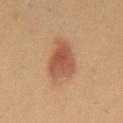| key | value |
|---|---|
| workup | no biopsy performed (imaged during a skin exam) |
| patient | female, aged approximately 40 |
| automated lesion analysis | a lesion–skin lightness drop of about 9 and a lesion-to-skin contrast of about 7.5 (normalized; higher = more distinct) |
| lesion size | ≈5.5 mm |
| site | the chest |
| image source | ~15 mm crop, total-body skin-cancer survey |
| tile lighting | cross-polarized |